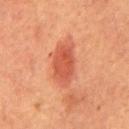workup — catalogued during a skin exam; not biopsied | imaging modality — ~15 mm tile from a whole-body skin photo | subject — male, roughly 65 years of age | anatomic site — the chest.